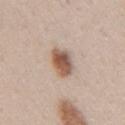No biopsy was performed on this lesion — it was imaged during a full skin examination and was not determined to be concerning.
On the right upper arm.
The subject is a female aged around 40.
The total-body-photography lesion software estimated a footprint of about 8 mm², an eccentricity of roughly 0.7, and a shape-asymmetry score of about 0.15 (0 = symmetric). The software also gave a lesion color around L≈56 a*≈18 b*≈28 in CIELAB and a lesion–skin lightness drop of about 15. And it measured a color-variation rating of about 5.5/10 and radial color variation of about 1.5. It also reported an automated nevus-likeness rating near 100 out of 100 and a lesion-detection confidence of about 100/100.
Longest diameter approximately 3.5 mm.
A roughly 15 mm field-of-view crop from a total-body skin photograph.
The tile uses white-light illumination.